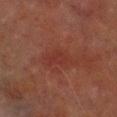biopsy_status: not biopsied; imaged during a skin examination
image:
  source: total-body photography crop
  field_of_view_mm: 15
site: right lower leg
lighting: cross-polarized
lesion_size:
  long_diameter_mm_approx: 4.5
patient:
  sex: male
  age_approx: 70